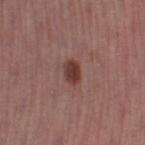  biopsy_status: not biopsied; imaged during a skin examination
  patient:
    sex: female
    age_approx: 55
  lighting: white-light
  image:
    source: total-body photography crop
    field_of_view_mm: 15
  automated_metrics:
    area_mm2_approx: 4.0
    eccentricity: 0.75
    shape_asymmetry: 0.15
    nevus_likeness_0_100: 95
    lesion_detection_confidence_0_100: 100
  lesion_size:
    long_diameter_mm_approx: 2.5
  site: left thigh